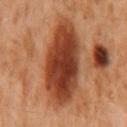The lesion was photographed on a routine skin check and not biopsied; there is no pathology result. Measured at roughly 9.5 mm in maximum diameter. Imaged with cross-polarized lighting. A lesion tile, about 15 mm wide, cut from a 3D total-body photograph. The lesion is located on the back. Automated image analysis of the tile measured a mean CIELAB color near L≈37 a*≈25 b*≈32. The analysis additionally found a classifier nevus-likeness of about 95/100 and a detector confidence of about 100 out of 100 that the crop contains a lesion. A male patient approximately 60 years of age.